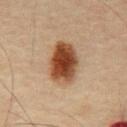Case summary:
• location — the chest
• lighting — cross-polarized illumination
• lesion size — ≈6 mm
• image source — ~15 mm tile from a whole-body skin photo
• patient — male, about 45 years old